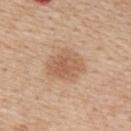Notes:
* notes · imaged on a skin check; not biopsied
* lesion size · ~4.5 mm (longest diameter)
* anatomic site · the right upper arm
* patient · male, in their 60s
* automated metrics · a peripheral color-asymmetry measure near 1; a classifier nevus-likeness of about 25/100 and a detector confidence of about 100 out of 100 that the crop contains a lesion
* tile lighting · white-light illumination
* image source · ~15 mm tile from a whole-body skin photo